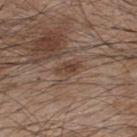notes — catalogued during a skin exam; not biopsied | location — the upper back | image — ~15 mm tile from a whole-body skin photo | subject — male, about 45 years old.